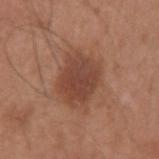Assessment:
No biopsy was performed on this lesion — it was imaged during a full skin examination and was not determined to be concerning.
Clinical summary:
A male subject aged around 35. A close-up tile cropped from a whole-body skin photograph, about 15 mm across. From the left upper arm.